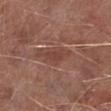Assessment: This lesion was catalogued during total-body skin photography and was not selected for biopsy. Clinical summary: The patient is a male in their mid-60s. From the leg. The tile uses white-light illumination. Cropped from a total-body skin-imaging series; the visible field is about 15 mm.